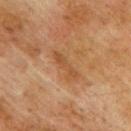The lesion is on the back. This is a cross-polarized tile. The lesion's longest dimension is about 4 mm. A 15 mm close-up tile from a total-body photography series done for melanoma screening. The patient is a male aged around 75.